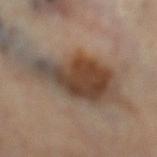Clinical impression: This lesion was catalogued during total-body skin photography and was not selected for biopsy. Context: A female subject aged 58 to 62. The recorded lesion diameter is about 11 mm. A 15 mm close-up extracted from a 3D total-body photography capture. Imaged with cross-polarized lighting. The lesion is on the left lower leg.